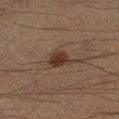follow-up = catalogued during a skin exam; not biopsied | patient = male, about 40 years old | size = ≈3.5 mm | illumination = cross-polarized | site = the left lower leg | acquisition = ~15 mm crop, total-body skin-cancer survey.